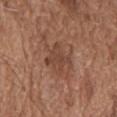patient = male, aged approximately 75
image source = 15 mm crop, total-body photography
body site = the chest